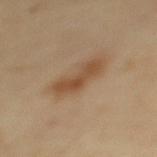Part of a total-body skin-imaging series; this lesion was reviewed on a skin check and was not flagged for biopsy. The lesion is located on the upper back. An algorithmic analysis of the crop reported border irregularity of about 4 on a 0–10 scale, internal color variation of about 3 on a 0–10 scale, and peripheral color asymmetry of about 1. It also reported an automated nevus-likeness rating near 85 out of 100 and a detector confidence of about 100 out of 100 that the crop contains a lesion. Approximately 4.5 mm at its widest. A female subject in their mid-60s. Imaged with cross-polarized lighting. A 15 mm crop from a total-body photograph taken for skin-cancer surveillance.